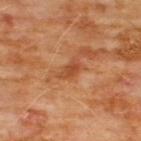* biopsy status — no biopsy performed (imaged during a skin exam)
* imaging modality — ~15 mm tile from a whole-body skin photo
* tile lighting — cross-polarized illumination
* subject — male, about 60 years old
* body site — the chest
* lesion size — ≈2.5 mm